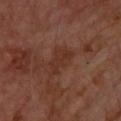Captured during whole-body skin photography for melanoma surveillance; the lesion was not biopsied.
This image is a 15 mm lesion crop taken from a total-body photograph.
Longest diameter approximately 3.5 mm.
From the upper back.
A male subject aged 68–72.
This is a cross-polarized tile.